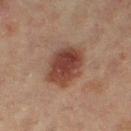notes: imaged on a skin check; not biopsied | anatomic site: the right thigh | TBP lesion metrics: a shape-asymmetry score of about 0.15 (0 = symmetric); a mean CIELAB color near L≈37 a*≈20 b*≈24, about 11 CIELAB-L* units darker than the surrounding skin, and a normalized lesion–skin contrast near 9.5; a nevus-likeness score of about 100/100 and a detector confidence of about 100 out of 100 that the crop contains a lesion | imaging modality: 15 mm crop, total-body photography | tile lighting: cross-polarized | diameter: about 6 mm | subject: female, aged around 55.